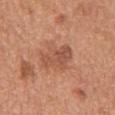Recorded during total-body skin imaging; not selected for excision or biopsy. A close-up tile cropped from a whole-body skin photograph, about 15 mm across. The subject is a male roughly 65 years of age. From the chest.